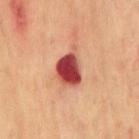This lesion was catalogued during total-body skin photography and was not selected for biopsy. A male subject approximately 70 years of age. Located on the front of the torso. A 15 mm close-up tile from a total-body photography series done for melanoma screening.